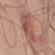On the left upper arm. Automated tile analysis of the lesion measured a footprint of about 18 mm², a shape eccentricity near 0.85, and a shape-asymmetry score of about 0.25 (0 = symmetric). It also reported a lesion color around L≈53 a*≈23 b*≈26 in CIELAB, roughly 9 lightness units darker than nearby skin, and a lesion-to-skin contrast of about 6.5 (normalized; higher = more distinct). It also reported an automated nevus-likeness rating near 5 out of 100 and lesion-presence confidence of about 95/100. Cropped from a whole-body photographic skin survey; the tile spans about 15 mm. Approximately 7.5 mm at its widest. Imaged with white-light lighting. The patient is a male roughly 50 years of age.